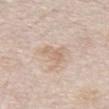The lesion was tiled from a total-body skin photograph and was not biopsied.
A 15 mm close-up extracted from a 3D total-body photography capture.
The lesion is on the front of the torso.
The subject is a male in their mid- to late 70s.